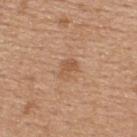This lesion was catalogued during total-body skin photography and was not selected for biopsy. A female patient in their mid-40s. On the upper back. Imaged with white-light lighting. A region of skin cropped from a whole-body photographic capture, roughly 15 mm wide. Approximately 2.5 mm at its widest.